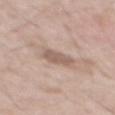location: the back
size: ≈3.5 mm
subject: male, roughly 50 years of age
acquisition: total-body-photography crop, ~15 mm field of view
lighting: white-light
automated metrics: an automated nevus-likeness rating near 0 out of 100 and a lesion-detection confidence of about 100/100